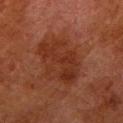Q: Was a biopsy performed?
A: total-body-photography surveillance lesion; no biopsy
Q: How large is the lesion?
A: ≈6.5 mm
Q: Lesion location?
A: the right lower leg
Q: Patient demographics?
A: male, aged approximately 80
Q: What kind of image is this?
A: total-body-photography crop, ~15 mm field of view